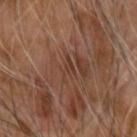– workup: catalogued during a skin exam; not biopsied
– image source: ~15 mm tile from a whole-body skin photo
– location: the right forearm
– image-analysis metrics: a footprint of about 3.5 mm², an outline eccentricity of about 0.9 (0 = round, 1 = elongated), and a symmetry-axis asymmetry near 0.5; a lesion color around L≈39 a*≈20 b*≈27 in CIELAB and a lesion–skin lightness drop of about 5; a border-irregularity rating of about 7/10 and a peripheral color-asymmetry measure near 0.5
– tile lighting: cross-polarized illumination
– diameter: about 4 mm
– subject: male, roughly 65 years of age